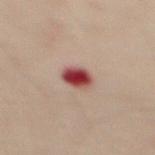follow-up = imaged on a skin check; not biopsied
tile lighting = cross-polarized
subject = male, about 50 years old
body site = the abdomen
image source = ~15 mm crop, total-body skin-cancer survey
lesion diameter = ~3.5 mm (longest diameter)